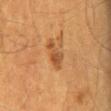Imaged during a routine full-body skin examination; the lesion was not biopsied and no histopathology is available. Imaged with cross-polarized lighting. Located on the mid back. The lesion-visualizer software estimated an area of roughly 4.5 mm², a shape eccentricity near 0.9, and a symmetry-axis asymmetry near 0.35. A male subject, aged 53 to 57. A lesion tile, about 15 mm wide, cut from a 3D total-body photograph. About 3.5 mm across.